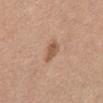{
  "biopsy_status": "not biopsied; imaged during a skin examination",
  "site": "abdomen",
  "lighting": "white-light",
  "image": {
    "source": "total-body photography crop",
    "field_of_view_mm": 15
  },
  "lesion_size": {
    "long_diameter_mm_approx": 2.5
  },
  "patient": {
    "sex": "female",
    "age_approx": 55
  }
}